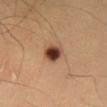| field | value |
|---|---|
| automated metrics | a lesion area of about 5.5 mm² and a symmetry-axis asymmetry near 0.2; a mean CIELAB color near L≈37 a*≈19 b*≈27, a lesion–skin lightness drop of about 17, and a normalized border contrast of about 13.5 |
| patient | male, aged 33 to 37 |
| acquisition | 15 mm crop, total-body photography |
| tile lighting | cross-polarized |
| site | the right lower leg |